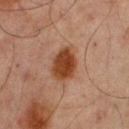biopsy_status: not biopsied; imaged during a skin examination
lesion_size:
  long_diameter_mm_approx: 4.0
lighting: cross-polarized
patient:
  sex: male
  age_approx: 65
image:
  source: total-body photography crop
  field_of_view_mm: 15
site: upper back